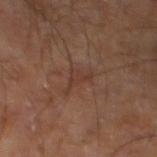subject: male, approximately 60 years of age
image source: ~15 mm tile from a whole-body skin photo
image-analysis metrics: a lesion area of about 3.5 mm², an eccentricity of roughly 0.85, and a symmetry-axis asymmetry near 0.6; a lesion color around L≈35 a*≈19 b*≈25 in CIELAB, about 5 CIELAB-L* units darker than the surrounding skin, and a lesion-to-skin contrast of about 5 (normalized; higher = more distinct); a border-irregularity rating of about 7.5/10, internal color variation of about 0.5 on a 0–10 scale, and radial color variation of about 0; an automated nevus-likeness rating near 0 out of 100 and a detector confidence of about 95 out of 100 that the crop contains a lesion
body site: the right leg
lesion diameter: about 3.5 mm
illumination: cross-polarized illumination